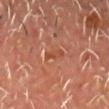– follow-up · total-body-photography surveillance lesion; no biopsy
– automated lesion analysis · internal color variation of about 0.5 on a 0–10 scale and peripheral color asymmetry of about 0
– lighting · cross-polarized illumination
– patient · male, roughly 60 years of age
– lesion size · ≈2.5 mm
– acquisition · ~15 mm tile from a whole-body skin photo